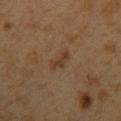Clinical impression: Imaged during a routine full-body skin examination; the lesion was not biopsied and no histopathology is available. Clinical summary: About 3.5 mm across. A female patient aged 38 to 42. The tile uses cross-polarized illumination. A 15 mm close-up tile from a total-body photography series done for melanoma screening. From the upper back.